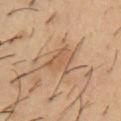Case summary:
• workup: no biopsy performed (imaged during a skin exam)
• image: ~15 mm tile from a whole-body skin photo
• patient: male, roughly 55 years of age
• location: the chest
• size: ~4 mm (longest diameter)